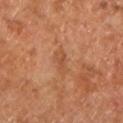{"biopsy_status": "not biopsied; imaged during a skin examination", "patient": {"age_approx": 65}, "lighting": "cross-polarized", "lesion_size": {"long_diameter_mm_approx": 3.0}, "automated_metrics": {"area_mm2_approx": 4.0, "shape_asymmetry": 0.25, "cielab_L": 50, "cielab_a": 24, "cielab_b": 36, "vs_skin_darker_L": 7.0, "vs_skin_contrast_norm": 5.0, "color_variation_0_10": 1.5, "nevus_likeness_0_100": 0}, "site": "left lower leg", "image": {"source": "total-body photography crop", "field_of_view_mm": 15}}